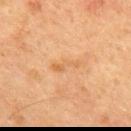Clinical impression:
The lesion was photographed on a routine skin check and not biopsied; there is no pathology result.
Acquisition and patient details:
Longest diameter approximately 3.5 mm. Cropped from a total-body skin-imaging series; the visible field is about 15 mm. A male subject aged around 60. Imaged with cross-polarized lighting.